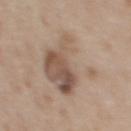Recorded during total-body skin imaging; not selected for excision or biopsy. A female patient, in their 50s. The total-body-photography lesion software estimated an area of roughly 28 mm², an outline eccentricity of about 0.65 (0 = round, 1 = elongated), and two-axis asymmetry of about 0.35. The analysis additionally found border irregularity of about 5.5 on a 0–10 scale, internal color variation of about 8 on a 0–10 scale, and peripheral color asymmetry of about 2.5. A 15 mm crop from a total-body photograph taken for skin-cancer surveillance. The lesion's longest dimension is about 7.5 mm. On the upper back. This is a white-light tile.